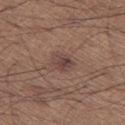This lesion was catalogued during total-body skin photography and was not selected for biopsy.
An algorithmic analysis of the crop reported a lesion area of about 5.5 mm² and an eccentricity of roughly 0.65. The software also gave an average lesion color of about L≈43 a*≈18 b*≈21 (CIELAB), a lesion–skin lightness drop of about 8, and a normalized border contrast of about 7. The software also gave a classifier nevus-likeness of about 30/100 and a detector confidence of about 100 out of 100 that the crop contains a lesion.
Located on the leg.
The patient is a male approximately 65 years of age.
A 15 mm close-up tile from a total-body photography series done for melanoma screening.
The tile uses white-light illumination.